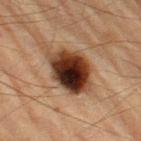{"biopsy_status": "not biopsied; imaged during a skin examination", "site": "leg", "lighting": "cross-polarized", "patient": {"sex": "male", "age_approx": "85+"}, "image": {"source": "total-body photography crop", "field_of_view_mm": 15}, "lesion_size": {"long_diameter_mm_approx": 6.5}}